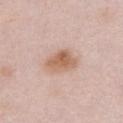<record>
  <image>
    <source>total-body photography crop</source>
    <field_of_view_mm>15</field_of_view_mm>
  </image>
  <site>chest</site>
  <patient>
    <sex>female</sex>
    <age_approx>65</age_approx>
  </patient>
  <automated_metrics>
    <border_irregularity_0_10>2.5</border_irregularity_0_10>
    <color_variation_0_10>4.0</color_variation_0_10>
    <peripheral_color_asymmetry>1.5</peripheral_color_asymmetry>
    <nevus_likeness_0_100>90</nevus_likeness_0_100>
    <lesion_detection_confidence_0_100>100</lesion_detection_confidence_0_100>
  </automated_metrics>
</record>